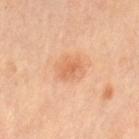| key | value |
|---|---|
| workup | no biopsy performed (imaged during a skin exam) |
| anatomic site | the right leg |
| size | ≈2.5 mm |
| patient | female, aged 58 to 62 |
| lighting | cross-polarized illumination |
| imaging modality | ~15 mm tile from a whole-body skin photo |